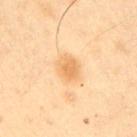The lesion was tiled from a total-body skin photograph and was not biopsied. Imaged with cross-polarized lighting. A roughly 15 mm field-of-view crop from a total-body skin photograph. From the right upper arm. The lesion's longest dimension is about 3 mm. A male subject aged 38 to 42.